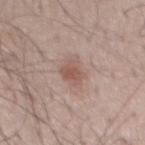This lesion was catalogued during total-body skin photography and was not selected for biopsy. Measured at roughly 3 mm in maximum diameter. A roughly 15 mm field-of-view crop from a total-body skin photograph. On the mid back. Automated image analysis of the tile measured an area of roughly 5.5 mm² and a shape eccentricity near 0.6. And it measured a lesion color around L≈54 a*≈19 b*≈24 in CIELAB and a normalized border contrast of about 6.5. The software also gave an automated nevus-likeness rating near 80 out of 100. This is a white-light tile. A male subject, aged approximately 50.